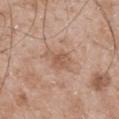notes = no biopsy performed (imaged during a skin exam); imaging modality = total-body-photography crop, ~15 mm field of view; lesion size = about 4 mm; site = the mid back; lighting = white-light illumination; subject = male, approximately 50 years of age.